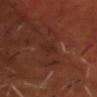follow-up: catalogued during a skin exam; not biopsied
site: the head or neck
size: about 2.5 mm
image: ~15 mm crop, total-body skin-cancer survey
illumination: cross-polarized
subject: male, aged 48–52